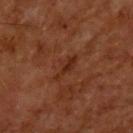Q: Was this lesion biopsied?
A: imaged on a skin check; not biopsied
Q: What kind of image is this?
A: ~15 mm tile from a whole-body skin photo
Q: Where on the body is the lesion?
A: the upper back
Q: What are the patient's age and sex?
A: male, in their mid- to late 60s
Q: What is the lesion's diameter?
A: about 4 mm